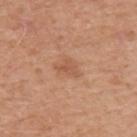No biopsy was performed on this lesion — it was imaged during a full skin examination and was not determined to be concerning. The lesion is on the back. Captured under white-light illumination. A lesion tile, about 15 mm wide, cut from a 3D total-body photograph. The lesion's longest dimension is about 2.5 mm. The patient is a male roughly 50 years of age.